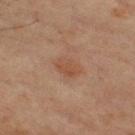tile lighting = cross-polarized illumination | size = about 3.5 mm | TBP lesion metrics = a footprint of about 6.5 mm², a shape eccentricity near 0.75, and a symmetry-axis asymmetry near 0.2; border irregularity of about 2 on a 0–10 scale, internal color variation of about 2 on a 0–10 scale, and a peripheral color-asymmetry measure near 0.5 | site = the leg | acquisition = ~15 mm tile from a whole-body skin photo | patient = male, roughly 60 years of age.